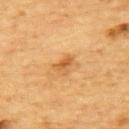Notes:
* lesion size · ~2.5 mm (longest diameter)
* subject · male, approximately 85 years of age or older
* acquisition · ~15 mm crop, total-body skin-cancer survey
* body site · the upper back
* illumination · cross-polarized
* image-analysis metrics · a border-irregularity rating of about 4/10, internal color variation of about 2 on a 0–10 scale, and radial color variation of about 0.5